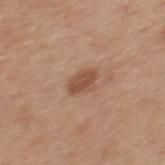Q: Was this lesion biopsied?
A: no biopsy performed (imaged during a skin exam)
Q: What is the anatomic site?
A: the mid back
Q: What did automated image analysis measure?
A: a mean CIELAB color near L≈50 a*≈21 b*≈31, about 11 CIELAB-L* units darker than the surrounding skin, and a normalized lesion–skin contrast near 7.5; an automated nevus-likeness rating near 75 out of 100 and lesion-presence confidence of about 100/100
Q: What are the patient's age and sex?
A: male, in their 30s
Q: How large is the lesion?
A: ~3 mm (longest diameter)
Q: What is the imaging modality?
A: 15 mm crop, total-body photography